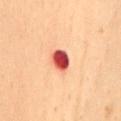Captured during whole-body skin photography for melanoma surveillance; the lesion was not biopsied. The subject is a female about 50 years old. From the back. Automated tile analysis of the lesion measured an area of roughly 5.5 mm², a shape eccentricity near 0.45, and a shape-asymmetry score of about 0.2 (0 = symmetric). Cropped from a total-body skin-imaging series; the visible field is about 15 mm. About 2.5 mm across.